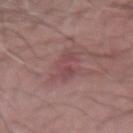– notes · no biopsy performed (imaged during a skin exam)
– acquisition · ~15 mm tile from a whole-body skin photo
– site · the right forearm
– patient · male, aged approximately 70
– size · ~4 mm (longest diameter)
– tile lighting · white-light illumination
– image-analysis metrics · a border-irregularity index near 5/10, a color-variation rating of about 3/10, and radial color variation of about 1; an automated nevus-likeness rating near 0 out of 100 and lesion-presence confidence of about 100/100